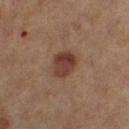image: ~15 mm tile from a whole-body skin photo; tile lighting: cross-polarized illumination; subject: female, in their 60s; diameter: ≈3 mm; location: the left thigh.